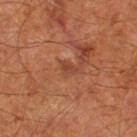Part of a total-body skin-imaging series; this lesion was reviewed on a skin check and was not flagged for biopsy. A male patient, roughly 65 years of age. A 15 mm close-up extracted from a 3D total-body photography capture. About 2 mm across. Imaged with cross-polarized lighting. On the left thigh.